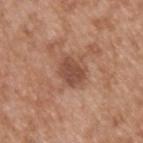The lesion was tiled from a total-body skin photograph and was not biopsied. Approximately 3 mm at its widest. From the upper back. The tile uses white-light illumination. A male patient in their mid- to late 60s. A 15 mm close-up tile from a total-body photography series done for melanoma screening.